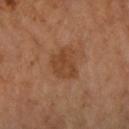This lesion was catalogued during total-body skin photography and was not selected for biopsy.
On the left forearm.
A region of skin cropped from a whole-body photographic capture, roughly 15 mm wide.
Captured under cross-polarized illumination.
About 3.5 mm across.
A female patient aged around 65.